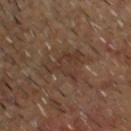Clinical impression: Part of a total-body skin-imaging series; this lesion was reviewed on a skin check and was not flagged for biopsy. Acquisition and patient details: The patient is a male approximately 60 years of age. The recorded lesion diameter is about 5.5 mm. On the head or neck. The tile uses cross-polarized illumination. A roughly 15 mm field-of-view crop from a total-body skin photograph.